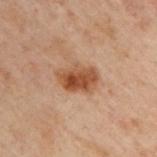Q: What lighting was used for the tile?
A: cross-polarized
Q: What did automated image analysis measure?
A: a normalized border contrast of about 9.5; a border-irregularity rating of about 2.5/10, a color-variation rating of about 5/10, and a peripheral color-asymmetry measure near 1.5
Q: Who is the patient?
A: male, in their 50s
Q: What kind of image is this?
A: ~15 mm tile from a whole-body skin photo
Q: What is the lesion's diameter?
A: ≈4 mm
Q: Where on the body is the lesion?
A: the upper back